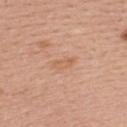Context: Captured under white-light illumination. Located on the upper back. A lesion tile, about 15 mm wide, cut from a 3D total-body photograph. The patient is a female about 40 years old.